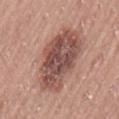Q: Was a biopsy performed?
A: no biopsy performed (imaged during a skin exam)
Q: How was this image acquired?
A: ~15 mm crop, total-body skin-cancer survey
Q: Automated lesion metrics?
A: an area of roughly 29 mm², an outline eccentricity of about 0.8 (0 = round, 1 = elongated), and a shape-asymmetry score of about 0.2 (0 = symmetric); a mean CIELAB color near L≈50 a*≈21 b*≈24 and about 14 CIELAB-L* units darker than the surrounding skin; a border-irregularity rating of about 2.5/10 and a color-variation rating of about 6.5/10; a classifier nevus-likeness of about 0/100 and lesion-presence confidence of about 100/100
Q: Lesion location?
A: the lower back
Q: What are the patient's age and sex?
A: male, approximately 20 years of age
Q: Illumination type?
A: white-light
Q: Lesion size?
A: ≈8 mm